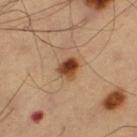biopsy_status: not biopsied; imaged during a skin examination
site: leg
image:
  source: total-body photography crop
  field_of_view_mm: 15
lesion_size:
  long_diameter_mm_approx: 2.5
patient:
  sex: male
  age_approx: 60